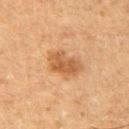Assessment:
Part of a total-body skin-imaging series; this lesion was reviewed on a skin check and was not flagged for biopsy.
Background:
A male subject, in their mid-70s. A region of skin cropped from a whole-body photographic capture, roughly 15 mm wide. From the left thigh. The tile uses cross-polarized illumination. Automated image analysis of the tile measured a lesion color around L≈46 a*≈19 b*≈33 in CIELAB and a normalized border contrast of about 7. The software also gave a border-irregularity rating of about 2.5/10, a within-lesion color-variation index near 3/10, and a peripheral color-asymmetry measure near 1. It also reported a classifier nevus-likeness of about 55/100 and a detector confidence of about 100 out of 100 that the crop contains a lesion.